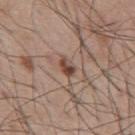biopsy status: imaged on a skin check; not biopsied
anatomic site: the mid back
TBP lesion metrics: a mean CIELAB color near L≈45 a*≈19 b*≈25 and about 12 CIELAB-L* units darker than the surrounding skin
image: ~15 mm crop, total-body skin-cancer survey
lesion size: about 2.5 mm
lighting: white-light
subject: male, approximately 55 years of age